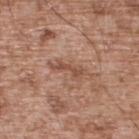Imaged during a routine full-body skin examination; the lesion was not biopsied and no histopathology is available. A lesion tile, about 15 mm wide, cut from a 3D total-body photograph. Located on the upper back. A male subject, in their mid-50s.